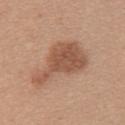Recorded during total-body skin imaging; not selected for excision or biopsy. A 15 mm close-up tile from a total-body photography series done for melanoma screening. Imaged with white-light lighting. The lesion is located on the upper back. A female patient aged 33 to 37.